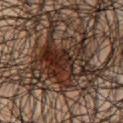workup: catalogued during a skin exam; not biopsied | location: the chest | subject: male, aged 63 to 67 | lighting: cross-polarized | size: ~9 mm (longest diameter) | image: 15 mm crop, total-body photography.